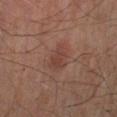Part of a total-body skin-imaging series; this lesion was reviewed on a skin check and was not flagged for biopsy. The lesion is located on the left lower leg. The patient is a male aged approximately 55. A 15 mm close-up tile from a total-body photography series done for melanoma screening.